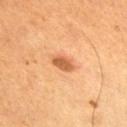image source: total-body-photography crop, ~15 mm field of view
TBP lesion metrics: a symmetry-axis asymmetry near 0.2; about 12 CIELAB-L* units darker than the surrounding skin and a normalized lesion–skin contrast near 8; border irregularity of about 2 on a 0–10 scale and a within-lesion color-variation index near 2/10; an automated nevus-likeness rating near 85 out of 100
size: about 3 mm
subject: male, in their mid- to late 50s
body site: the front of the torso